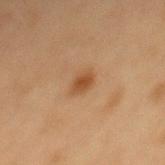follow-up: imaged on a skin check; not biopsied
subject: female, aged around 40
lesion diameter: ~3 mm (longest diameter)
illumination: cross-polarized illumination
acquisition: total-body-photography crop, ~15 mm field of view
anatomic site: the mid back